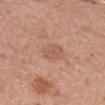Q: Is there a histopathology result?
A: no biopsy performed (imaged during a skin exam)
Q: What kind of image is this?
A: ~15 mm tile from a whole-body skin photo
Q: What are the patient's age and sex?
A: female, in their mid- to late 30s
Q: How was the tile lit?
A: white-light illumination
Q: What is the anatomic site?
A: the left forearm